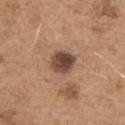The lesion was photographed on a routine skin check and not biopsied; there is no pathology result. Longest diameter approximately 3 mm. The lesion is located on the chest. This is a white-light tile. A 15 mm crop from a total-body photograph taken for skin-cancer surveillance. A male subject, about 65 years old. The total-body-photography lesion software estimated an area of roughly 7.5 mm², an eccentricity of roughly 0.4, and two-axis asymmetry of about 0.15. The analysis additionally found a within-lesion color-variation index near 5.5/10.